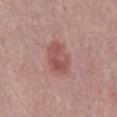The lesion was photographed on a routine skin check and not biopsied; there is no pathology result.
The lesion is located on the abdomen.
Cropped from a whole-body photographic skin survey; the tile spans about 15 mm.
A male subject aged 63 to 67.